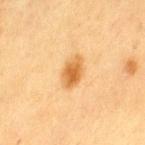{"biopsy_status": "not biopsied; imaged during a skin examination"}